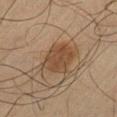biopsy status: no biopsy performed (imaged during a skin exam) | size: about 4 mm | subject: male, aged 63 to 67 | acquisition: total-body-photography crop, ~15 mm field of view | site: the leg | lighting: cross-polarized illumination | image-analysis metrics: an average lesion color of about L≈36 a*≈15 b*≈26 (CIELAB), about 8 CIELAB-L* units darker than the surrounding skin, and a lesion-to-skin contrast of about 7.5 (normalized; higher = more distinct); a color-variation rating of about 2/10 and a peripheral color-asymmetry measure near 1; a classifier nevus-likeness of about 50/100.